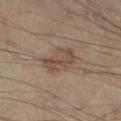The lesion was photographed on a routine skin check and not biopsied; there is no pathology result. On the leg. A male patient approximately 55 years of age. A roughly 15 mm field-of-view crop from a total-body skin photograph. Approximately 4.5 mm at its widest.